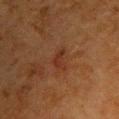Recorded during total-body skin imaging; not selected for excision or biopsy. The patient is a female in their mid-50s. The tile uses cross-polarized illumination. On the back. Cropped from a whole-body photographic skin survey; the tile spans about 15 mm.